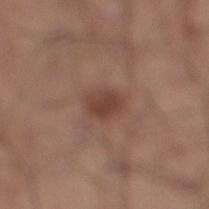Recorded during total-body skin imaging; not selected for excision or biopsy. Located on the right lower leg. Longest diameter approximately 3 mm. The patient is a male about 35 years old. A lesion tile, about 15 mm wide, cut from a 3D total-body photograph. This is a white-light tile. The total-body-photography lesion software estimated a footprint of about 5 mm², a shape eccentricity near 0.7, and two-axis asymmetry of about 0.25. It also reported an automated nevus-likeness rating near 85 out of 100 and a lesion-detection confidence of about 100/100.